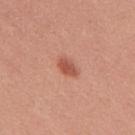<record>
  <biopsy_status>not biopsied; imaged during a skin examination</biopsy_status>
  <patient>
    <sex>female</sex>
    <age_approx>25</age_approx>
  </patient>
  <site>upper back</site>
  <image>
    <source>total-body photography crop</source>
    <field_of_view_mm>15</field_of_view_mm>
  </image>
</record>